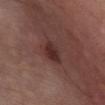| key | value |
|---|---|
| notes | imaged on a skin check; not biopsied |
| image source | ~15 mm tile from a whole-body skin photo |
| anatomic site | the chest |
| patient | male, aged around 75 |